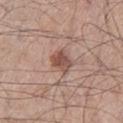follow-up = imaged on a skin check; not biopsied
image source = ~15 mm crop, total-body skin-cancer survey
body site = the leg
patient = male, aged 53–57
lighting = white-light